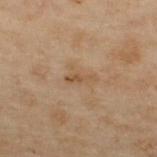This lesion was catalogued during total-body skin photography and was not selected for biopsy. Approximately 3 mm at its widest. This is a cross-polarized tile. From the upper back. This image is a 15 mm lesion crop taken from a total-body photograph. Automated tile analysis of the lesion measured an area of roughly 2.5 mm², an outline eccentricity of about 0.95 (0 = round, 1 = elongated), and a shape-asymmetry score of about 0.45 (0 = symmetric). The software also gave a border-irregularity index near 5.5/10, internal color variation of about 0 on a 0–10 scale, and peripheral color asymmetry of about 0. A male subject aged approximately 50.